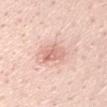Imaged during a routine full-body skin examination; the lesion was not biopsied and no histopathology is available.
An algorithmic analysis of the crop reported a footprint of about 7 mm², a shape eccentricity near 0.65, and two-axis asymmetry of about 0.2. The software also gave a lesion color around L≈70 a*≈23 b*≈27 in CIELAB, a lesion–skin lightness drop of about 10, and a lesion-to-skin contrast of about 6 (normalized; higher = more distinct).
A close-up tile cropped from a whole-body skin photograph, about 15 mm across.
The lesion is on the right upper arm.
A male patient, roughly 50 years of age.
The recorded lesion diameter is about 3.5 mm.
Imaged with white-light lighting.